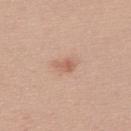• biopsy status · imaged on a skin check; not biopsied
• acquisition · total-body-photography crop, ~15 mm field of view
• illumination · white-light
• subject · male, about 30 years old
• diameter · about 2.5 mm